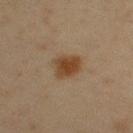The lesion was photographed on a routine skin check and not biopsied; there is no pathology result.
The lesion is located on the right upper arm.
A close-up tile cropped from a whole-body skin photograph, about 15 mm across.
The subject is a female aged approximately 45.